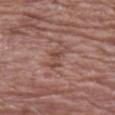Notes:
- follow-up — no biopsy performed (imaged during a skin exam)
- subject — female, about 70 years old
- image — ~15 mm crop, total-body skin-cancer survey
- body site — the arm
- lesion size — ~3.5 mm (longest diameter)